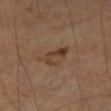Clinical impression:
Imaged during a routine full-body skin examination; the lesion was not biopsied and no histopathology is available.
Clinical summary:
From the left thigh. A male subject, in their 70s. A lesion tile, about 15 mm wide, cut from a 3D total-body photograph.